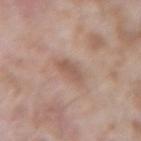The lesion was photographed on a routine skin check and not biopsied; there is no pathology result.
The lesion is on the left forearm.
The total-body-photography lesion software estimated an eccentricity of roughly 0.8 and a shape-asymmetry score of about 0.3 (0 = symmetric). And it measured a lesion–skin lightness drop of about 8. It also reported a border-irregularity rating of about 3/10 and a within-lesion color-variation index near 2/10. And it measured a classifier nevus-likeness of about 0/100 and a lesion-detection confidence of about 100/100.
The tile uses white-light illumination.
Measured at roughly 3 mm in maximum diameter.
A male patient, aged around 60.
A region of skin cropped from a whole-body photographic capture, roughly 15 mm wide.